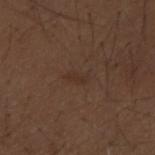follow-up: imaged on a skin check; not biopsied
site: the back
patient: male, aged 48–52
lighting: white-light illumination
image: ~15 mm tile from a whole-body skin photo
TBP lesion metrics: an area of roughly 3 mm² and a shape-asymmetry score of about 0.35 (0 = symmetric); a lesion color around L≈31 a*≈16 b*≈24 in CIELAB, roughly 4 lightness units darker than nearby skin, and a lesion-to-skin contrast of about 4.5 (normalized; higher = more distinct)